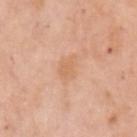Impression: Imaged during a routine full-body skin examination; the lesion was not biopsied and no histopathology is available. Background: The subject is a female aged approximately 65. A 15 mm close-up tile from a total-body photography series done for melanoma screening. On the left upper arm.